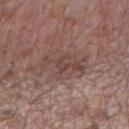| key | value |
|---|---|
| follow-up | total-body-photography surveillance lesion; no biopsy |
| acquisition | total-body-photography crop, ~15 mm field of view |
| patient | male, about 70 years old |
| lighting | white-light illumination |
| body site | the mid back |